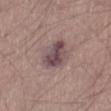- workup — total-body-photography surveillance lesion; no biopsy
- automated metrics — an average lesion color of about L≈47 a*≈17 b*≈14 (CIELAB) and roughly 11 lightness units darker than nearby skin; a nevus-likeness score of about 25/100
- imaging modality — ~15 mm crop, total-body skin-cancer survey
- patient — male, aged approximately 65
- location — the right thigh